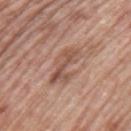follow-up — catalogued during a skin exam; not biopsied | subject — male, in their 70s | imaging modality — total-body-photography crop, ~15 mm field of view | anatomic site — the left upper arm.